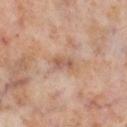Impression: Part of a total-body skin-imaging series; this lesion was reviewed on a skin check and was not flagged for biopsy. Image and clinical context: On the right lower leg. Longest diameter approximately 2.5 mm. A 15 mm crop from a total-body photograph taken for skin-cancer surveillance. A female patient, approximately 55 years of age. Imaged with cross-polarized lighting.